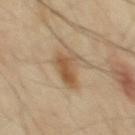Assessment:
Imaged during a routine full-body skin examination; the lesion was not biopsied and no histopathology is available.
Image and clinical context:
The lesion is located on the mid back. A lesion tile, about 15 mm wide, cut from a 3D total-body photograph. An algorithmic analysis of the crop reported a lesion area of about 10 mm², a shape eccentricity near 0.8, and a symmetry-axis asymmetry near 0.45. The software also gave a border-irregularity rating of about 4.5/10, internal color variation of about 5.5 on a 0–10 scale, and radial color variation of about 2. The software also gave an automated nevus-likeness rating near 90 out of 100 and lesion-presence confidence of about 100/100. A male patient, aged around 55. Imaged with cross-polarized lighting.